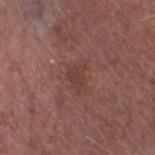Q: Is there a histopathology result?
A: imaged on a skin check; not biopsied
Q: Automated lesion metrics?
A: a lesion area of about 4 mm², a shape eccentricity near 0.75, and two-axis asymmetry of about 0.4
Q: How was the tile lit?
A: white-light illumination
Q: What kind of image is this?
A: 15 mm crop, total-body photography
Q: What is the anatomic site?
A: the left thigh
Q: Who is the patient?
A: male, roughly 75 years of age